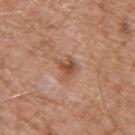Part of a total-body skin-imaging series; this lesion was reviewed on a skin check and was not flagged for biopsy. A male patient, aged 78 to 82. A close-up tile cropped from a whole-body skin photograph, about 15 mm across. The tile uses white-light illumination. From the upper back. About 2.5 mm across. Automated image analysis of the tile measured a lesion area of about 4.5 mm² and an outline eccentricity of about 0.5 (0 = round, 1 = elongated). It also reported a lesion color around L≈51 a*≈22 b*≈31 in CIELAB, roughly 10 lightness units darker than nearby skin, and a lesion-to-skin contrast of about 7.5 (normalized; higher = more distinct). It also reported border irregularity of about 4 on a 0–10 scale. And it measured a classifier nevus-likeness of about 15/100.